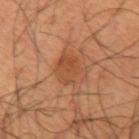Findings:
* notes — total-body-photography surveillance lesion; no biopsy
* lesion size — ~4 mm (longest diameter)
* location — the left upper arm
* imaging modality — 15 mm crop, total-body photography
* automated lesion analysis — an area of roughly 10 mm², an eccentricity of roughly 0.65, and two-axis asymmetry of about 0.3; border irregularity of about 3.5 on a 0–10 scale and radial color variation of about 1.5; a nevus-likeness score of about 65/100
* patient — male, roughly 40 years of age